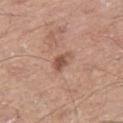<record>
  <biopsy_status>not biopsied; imaged during a skin examination</biopsy_status>
  <site>right thigh</site>
  <patient>
    <sex>male</sex>
    <age_approx>60</age_approx>
  </patient>
  <lesion_size>
    <long_diameter_mm_approx>2.5</long_diameter_mm_approx>
  </lesion_size>
  <image>
    <source>total-body photography crop</source>
    <field_of_view_mm>15</field_of_view_mm>
  </image>
  <lighting>white-light</lighting>
</record>